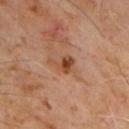workup = catalogued during a skin exam; not biopsied
image source = ~15 mm tile from a whole-body skin photo
subject = male, about 60 years old
site = the chest